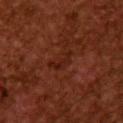Assessment: Captured during whole-body skin photography for melanoma surveillance; the lesion was not biopsied. Context: The total-body-photography lesion software estimated a lesion area of about 3.5 mm², an eccentricity of roughly 0.9, and two-axis asymmetry of about 0.65. The analysis additionally found a nevus-likeness score of about 80/100. The lesion is on the back. This is a cross-polarized tile. A 15 mm crop from a total-body photograph taken for skin-cancer surveillance. The subject is a male aged around 45. Longest diameter approximately 3.5 mm.